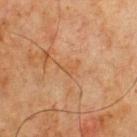Notes:
• workup — imaged on a skin check; not biopsied
• image source — ~15 mm crop, total-body skin-cancer survey
• site — the chest
• illumination — cross-polarized
• subject — male, aged around 65
• size — ~2.5 mm (longest diameter)